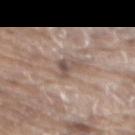This lesion was catalogued during total-body skin photography and was not selected for biopsy. From the chest. The subject is a male roughly 80 years of age. The tile uses white-light illumination. Longest diameter approximately 3.5 mm. The lesion-visualizer software estimated a lesion color around L≈52 a*≈14 b*≈22 in CIELAB and roughly 9 lightness units darker than nearby skin. It also reported border irregularity of about 5 on a 0–10 scale and a color-variation rating of about 5/10. And it measured a nevus-likeness score of about 0/100. Cropped from a total-body skin-imaging series; the visible field is about 15 mm.